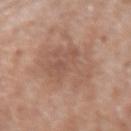Assessment: Part of a total-body skin-imaging series; this lesion was reviewed on a skin check and was not flagged for biopsy. Context: Imaged with white-light lighting. The recorded lesion diameter is about 7.5 mm. A female subject, roughly 70 years of age. A 15 mm close-up tile from a total-body photography series done for melanoma screening. The lesion is on the right forearm.